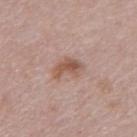The lesion was tiled from a total-body skin photograph and was not biopsied. Automated tile analysis of the lesion measured a lesion area of about 7 mm², an eccentricity of roughly 0.75, and a symmetry-axis asymmetry near 0.3. It also reported a border-irregularity index near 3/10 and internal color variation of about 4.5 on a 0–10 scale. The analysis additionally found an automated nevus-likeness rating near 65 out of 100 and a lesion-detection confidence of about 100/100. A 15 mm close-up extracted from a 3D total-body photography capture. The subject is a male aged around 75. On the abdomen. Measured at roughly 3.5 mm in maximum diameter.